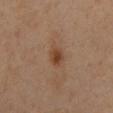Imaged during a routine full-body skin examination; the lesion was not biopsied and no histopathology is available.